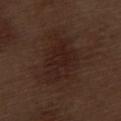Context:
A male patient, approximately 70 years of age. This image is a 15 mm lesion crop taken from a total-body photograph. The lesion is on the left thigh.